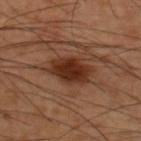{"biopsy_status": "not biopsied; imaged during a skin examination", "site": "upper back", "lighting": "cross-polarized", "patient": {"sex": "male", "age_approx": 60}, "image": {"source": "total-body photography crop", "field_of_view_mm": 15}, "automated_metrics": {"area_mm2_approx": 13.0, "eccentricity": 0.75, "shape_asymmetry": 0.2, "nevus_likeness_0_100": 95}}